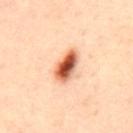Acquisition and patient details: The recorded lesion diameter is about 4.5 mm. The subject is a male in their mid-30s. Captured under cross-polarized illumination. This image is a 15 mm lesion crop taken from a total-body photograph. The lesion is on the mid back. Automated tile analysis of the lesion measured a shape eccentricity near 0.85 and a symmetry-axis asymmetry near 0.2. The analysis additionally found a lesion color around L≈62 a*≈29 b*≈38 in CIELAB and a lesion-to-skin contrast of about 12.5 (normalized; higher = more distinct). It also reported a nevus-likeness score of about 100/100. Diagnosis: Histopathology of the biopsied lesion showed a compound melanocytic nevus (benign).